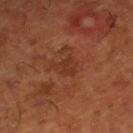Findings:
• workup: total-body-photography surveillance lesion; no biopsy
• lesion size: ~3 mm (longest diameter)
• image: ~15 mm crop, total-body skin-cancer survey
• patient: male, aged approximately 65
• location: the right lower leg
• tile lighting: cross-polarized illumination